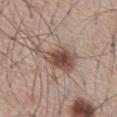Imaged during a routine full-body skin examination; the lesion was not biopsied and no histopathology is available.
The lesion is on the chest.
A male patient, aged around 65.
The lesion's longest dimension is about 5.5 mm.
Captured under white-light illumination.
This image is a 15 mm lesion crop taken from a total-body photograph.